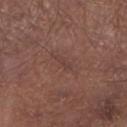Findings:
- biopsy status · total-body-photography surveillance lesion; no biopsy
- tile lighting · white-light
- body site · the left lower leg
- subject · male, aged approximately 55
- imaging modality · ~15 mm crop, total-body skin-cancer survey